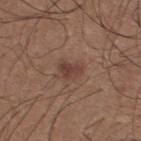Clinical impression:
Captured during whole-body skin photography for melanoma surveillance; the lesion was not biopsied.
Background:
This image is a 15 mm lesion crop taken from a total-body photograph. An algorithmic analysis of the crop reported a mean CIELAB color near L≈40 a*≈19 b*≈24, roughly 8 lightness units darker than nearby skin, and a normalized border contrast of about 7. A male patient, aged approximately 25. The recorded lesion diameter is about 2.5 mm. This is a white-light tile. From the upper back.